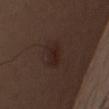Captured during whole-body skin photography for melanoma surveillance; the lesion was not biopsied.
Captured under white-light illumination.
The lesion's longest dimension is about 3.5 mm.
A male patient, aged approximately 30.
A close-up tile cropped from a whole-body skin photograph, about 15 mm across.
From the chest.